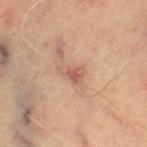biopsy_status: not biopsied; imaged during a skin examination
site: right thigh
image:
  source: total-body photography crop
  field_of_view_mm: 15
patient:
  sex: male
  age_approx: 70
lighting: cross-polarized
lesion_size:
  long_diameter_mm_approx: 3.0